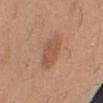follow-up: total-body-photography surveillance lesion; no biopsy
lesion size: about 4 mm
patient: male, about 40 years old
automated metrics: a color-variation rating of about 2/10 and peripheral color asymmetry of about 1; an automated nevus-likeness rating near 35 out of 100 and a lesion-detection confidence of about 100/100
location: the right upper arm
lighting: white-light illumination
image source: ~15 mm tile from a whole-body skin photo